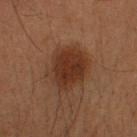biopsy status: imaged on a skin check; not biopsied | imaging modality: total-body-photography crop, ~15 mm field of view | lighting: cross-polarized illumination | body site: the head or neck | patient: male, roughly 35 years of age | size: ~5 mm (longest diameter) | TBP lesion metrics: a lesion color around L≈29 a*≈19 b*≈26 in CIELAB; border irregularity of about 2 on a 0–10 scale, a color-variation rating of about 3.5/10, and peripheral color asymmetry of about 1.